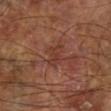follow-up — no biopsy performed (imaged during a skin exam)
patient — male, aged 58–62
imaging modality — 15 mm crop, total-body photography
image-analysis metrics — an area of roughly 6 mm², an eccentricity of roughly 0.55, and a shape-asymmetry score of about 0.3 (0 = symmetric); a lesion color around L≈36 a*≈23 b*≈26 in CIELAB, a lesion–skin lightness drop of about 6, and a normalized lesion–skin contrast near 5.5; a border-irregularity rating of about 3.5/10, a within-lesion color-variation index near 2.5/10, and a peripheral color-asymmetry measure near 1
location — the left lower leg
lighting — cross-polarized illumination
size — ≈3 mm